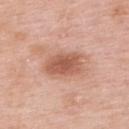Q: Was this lesion biopsied?
A: no biopsy performed (imaged during a skin exam)
Q: Illumination type?
A: white-light illumination
Q: Patient demographics?
A: female, aged around 50
Q: What is the lesion's diameter?
A: about 4.5 mm
Q: What kind of image is this?
A: ~15 mm crop, total-body skin-cancer survey
Q: What is the anatomic site?
A: the upper back